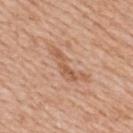No biopsy was performed on this lesion — it was imaged during a full skin examination and was not determined to be concerning.
The patient is a female aged 48 to 52.
The total-body-photography lesion software estimated a lesion color around L≈58 a*≈22 b*≈33 in CIELAB, roughly 8 lightness units darker than nearby skin, and a normalized border contrast of about 6.
Located on the upper back.
A roughly 15 mm field-of-view crop from a total-body skin photograph.
The recorded lesion diameter is about 6 mm.